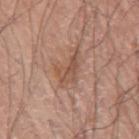This lesion was catalogued during total-body skin photography and was not selected for biopsy.
A region of skin cropped from a whole-body photographic capture, roughly 15 mm wide.
The lesion's longest dimension is about 4.5 mm.
A male subject, in their 70s.
Imaged with white-light lighting.
From the left arm.